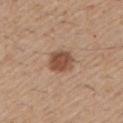The lesion was tiled from a total-body skin photograph and was not biopsied. Measured at roughly 3.5 mm in maximum diameter. A male subject aged 58 to 62. On the left upper arm. Captured under white-light illumination. The total-body-photography lesion software estimated a lesion area of about 7.5 mm², a shape eccentricity near 0.5, and two-axis asymmetry of about 0.2. The analysis additionally found border irregularity of about 1.5 on a 0–10 scale and internal color variation of about 3 on a 0–10 scale. A roughly 15 mm field-of-view crop from a total-body skin photograph.